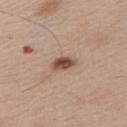Imaged during a routine full-body skin examination; the lesion was not biopsied and no histopathology is available.
On the chest.
The lesion's longest dimension is about 3 mm.
A 15 mm close-up tile from a total-body photography series done for melanoma screening.
A male subject, about 65 years old.